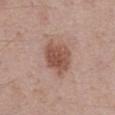biopsy status: no biopsy performed (imaged during a skin exam)
lesion diameter: ~4.5 mm (longest diameter)
TBP lesion metrics: a mean CIELAB color near L≈51 a*≈21 b*≈26, roughly 12 lightness units darker than nearby skin, and a lesion-to-skin contrast of about 8.5 (normalized; higher = more distinct); a border-irregularity index near 2/10, a within-lesion color-variation index near 4/10, and radial color variation of about 1.5; an automated nevus-likeness rating near 90 out of 100
subject: male, aged 68–72
lighting: white-light
acquisition: total-body-photography crop, ~15 mm field of view
site: the front of the torso